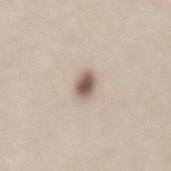biopsy status: catalogued during a skin exam; not biopsied | imaging modality: ~15 mm crop, total-body skin-cancer survey | anatomic site: the mid back | lesion diameter: ~2.5 mm (longest diameter) | patient: female, roughly 40 years of age | illumination: white-light illumination.